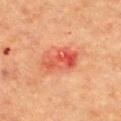Case summary:
- follow-up: imaged on a skin check; not biopsied
- tile lighting: cross-polarized
- TBP lesion metrics: an average lesion color of about L≈51 a*≈34 b*≈34 (CIELAB), roughly 10 lightness units darker than nearby skin, and a normalized border contrast of about 7; a border-irregularity rating of about 3/10, a within-lesion color-variation index near 9/10, and a peripheral color-asymmetry measure near 4
- anatomic site: the chest
- imaging modality: ~15 mm tile from a whole-body skin photo
- patient: female, aged 58–62
- diameter: about 4.5 mm